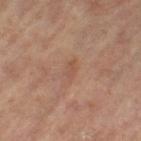{"biopsy_status": "not biopsied; imaged during a skin examination", "automated_metrics": {"eccentricity": 0.9, "shape_asymmetry": 0.4, "cielab_L": 49, "cielab_a": 20, "cielab_b": 28, "vs_skin_darker_L": 6.0, "vs_skin_contrast_norm": 4.5, "border_irregularity_0_10": 3.5, "color_variation_0_10": 1.0}, "lesion_size": {"long_diameter_mm_approx": 2.5}, "patient": {"sex": "female", "age_approx": 65}, "lighting": "cross-polarized", "site": "right leg", "image": {"source": "total-body photography crop", "field_of_view_mm": 15}}